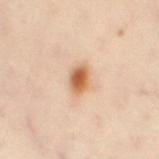The lesion was photographed on a routine skin check and not biopsied; there is no pathology result. A female subject in their 40s. A 15 mm close-up extracted from a 3D total-body photography capture. The lesion is located on the leg.